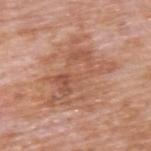Impression: This lesion was catalogued during total-body skin photography and was not selected for biopsy. Image and clinical context: About 7.5 mm across. The subject is a male aged around 60. Automated tile analysis of the lesion measured a border-irregularity index near 8/10, a within-lesion color-variation index near 5.5/10, and a peripheral color-asymmetry measure near 2. The software also gave a detector confidence of about 100 out of 100 that the crop contains a lesion. On the upper back. A lesion tile, about 15 mm wide, cut from a 3D total-body photograph. The tile uses white-light illumination.